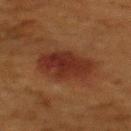Recorded during total-body skin imaging; not selected for excision or biopsy.
This is a cross-polarized tile.
Approximately 5.5 mm at its widest.
A female subject, in their 50s.
Located on the upper back.
A region of skin cropped from a whole-body photographic capture, roughly 15 mm wide.